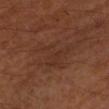{
  "biopsy_status": "not biopsied; imaged during a skin examination",
  "automated_metrics": {
    "area_mm2_approx": 15.0,
    "border_irregularity_0_10": 9.0,
    "color_variation_0_10": 1.5,
    "nevus_likeness_0_100": 0,
    "lesion_detection_confidence_0_100": 95
  },
  "image": {
    "source": "total-body photography crop",
    "field_of_view_mm": 15
  },
  "patient": {
    "sex": "male",
    "age_approx": 50
  },
  "site": "left forearm"
}